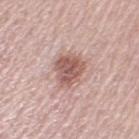The lesion was tiled from a total-body skin photograph and was not biopsied. Imaged with white-light lighting. A 15 mm crop from a total-body photograph taken for skin-cancer surveillance. An algorithmic analysis of the crop reported an average lesion color of about L≈57 a*≈21 b*≈23 (CIELAB), a lesion–skin lightness drop of about 13, and a lesion-to-skin contrast of about 8.5 (normalized; higher = more distinct). It also reported an automated nevus-likeness rating near 70 out of 100 and a detector confidence of about 100 out of 100 that the crop contains a lesion. A female subject aged around 65. Located on the left upper arm.